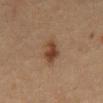Imaged during a routine full-body skin examination; the lesion was not biopsied and no histopathology is available.
Automated image analysis of the tile measured a lesion area of about 6 mm² and a shape-asymmetry score of about 0.3 (0 = symmetric). It also reported a lesion–skin lightness drop of about 9 and a lesion-to-skin contrast of about 8.5 (normalized; higher = more distinct). And it measured a border-irregularity rating of about 3/10, a color-variation rating of about 3.5/10, and radial color variation of about 1. And it measured lesion-presence confidence of about 100/100.
A close-up tile cropped from a whole-body skin photograph, about 15 mm across.
A male patient about 65 years old.
The lesion is on the mid back.